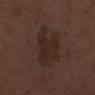The lesion was photographed on a routine skin check and not biopsied; there is no pathology result. From the right lower leg. A 15 mm crop from a total-body photograph taken for skin-cancer surveillance. The subject is a male aged around 70. Automated tile analysis of the lesion measured a footprint of about 18 mm² and an eccentricity of roughly 0.75. The software also gave a lesion–skin lightness drop of about 6 and a lesion-to-skin contrast of about 6.5 (normalized; higher = more distinct). It also reported a border-irregularity index near 3.5/10, a within-lesion color-variation index near 2/10, and a peripheral color-asymmetry measure near 1. This is a white-light tile. About 6 mm across.